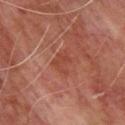notes: catalogued during a skin exam; not biopsied